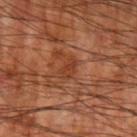follow-up: total-body-photography surveillance lesion; no biopsy
patient: male, aged 58 to 62
TBP lesion metrics: a lesion area of about 3.5 mm², an eccentricity of roughly 0.85, and two-axis asymmetry of about 0.25; roughly 7 lightness units darker than nearby skin and a normalized lesion–skin contrast near 6
location: the left upper arm
size: ~2.5 mm (longest diameter)
acquisition: total-body-photography crop, ~15 mm field of view